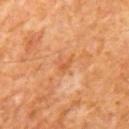Image and clinical context: Automated tile analysis of the lesion measured an average lesion color of about L≈57 a*≈27 b*≈41 (CIELAB) and a lesion-to-skin contrast of about 4.5 (normalized; higher = more distinct). The analysis additionally found a classifier nevus-likeness of about 0/100 and a lesion-detection confidence of about 100/100. This is a cross-polarized tile. Approximately 3 mm at its widest. A region of skin cropped from a whole-body photographic capture, roughly 15 mm wide. A male patient aged 63–67. The lesion is on the mid back.